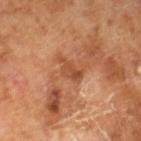biopsy_status: not biopsied; imaged during a skin examination
image:
  source: total-body photography crop
  field_of_view_mm: 15
lighting: cross-polarized
lesion_size:
  long_diameter_mm_approx: 3.5
patient:
  sex: male
  age_approx: 65